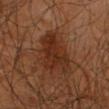Part of a total-body skin-imaging series; this lesion was reviewed on a skin check and was not flagged for biopsy. Imaged with cross-polarized lighting. Located on the right upper arm. Cropped from a whole-body photographic skin survey; the tile spans about 15 mm. A male subject in their mid-60s. Measured at roughly 5 mm in maximum diameter. An algorithmic analysis of the crop reported a lesion area of about 15 mm² and two-axis asymmetry of about 0.4. It also reported roughly 8 lightness units darker than nearby skin and a lesion-to-skin contrast of about 8.5 (normalized; higher = more distinct). And it measured a border-irregularity rating of about 4/10, a color-variation rating of about 3.5/10, and peripheral color asymmetry of about 1. It also reported an automated nevus-likeness rating near 5 out of 100 and lesion-presence confidence of about 100/100.